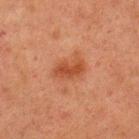Imaged during a routine full-body skin examination; the lesion was not biopsied and no histopathology is available. On the upper back. The tile uses cross-polarized illumination. A lesion tile, about 15 mm wide, cut from a 3D total-body photograph. Longest diameter approximately 4 mm. A male subject, roughly 60 years of age.